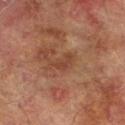Imaged during a routine full-body skin examination; the lesion was not biopsied and no histopathology is available. Approximately 3.5 mm at its widest. The lesion is located on the leg. A close-up tile cropped from a whole-body skin photograph, about 15 mm across. The tile uses cross-polarized illumination. The subject is a male about 75 years old.